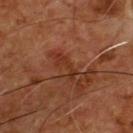The lesion was tiled from a total-body skin photograph and was not biopsied. A male subject aged around 55. Longest diameter approximately 3.5 mm. The tile uses cross-polarized illumination. A close-up tile cropped from a whole-body skin photograph, about 15 mm across. On the front of the torso.